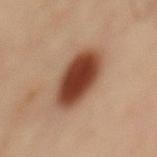This lesion was catalogued during total-body skin photography and was not selected for biopsy. The lesion-visualizer software estimated a lesion area of about 19 mm² and an outline eccentricity of about 0.75 (0 = round, 1 = elongated). It also reported a detector confidence of about 100 out of 100 that the crop contains a lesion. Imaged with cross-polarized lighting. Located on the back. Longest diameter approximately 6 mm. The subject is a male aged 48 to 52. A region of skin cropped from a whole-body photographic capture, roughly 15 mm wide.